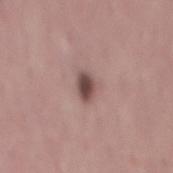The recorded lesion diameter is about 3 mm.
An algorithmic analysis of the crop reported a lesion area of about 4.5 mm², a shape eccentricity near 0.8, and a shape-asymmetry score of about 0.2 (0 = symmetric). And it measured an automated nevus-likeness rating near 95 out of 100 and lesion-presence confidence of about 100/100.
From the back.
This is a white-light tile.
A 15 mm crop from a total-body photograph taken for skin-cancer surveillance.
The subject is a male in their 50s.